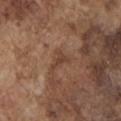<tbp_lesion>
  <biopsy_status>not biopsied; imaged during a skin examination</biopsy_status>
  <lesion_size>
    <long_diameter_mm_approx>3.0</long_diameter_mm_approx>
  </lesion_size>
  <lighting>white-light</lighting>
  <image>
    <source>total-body photography crop</source>
    <field_of_view_mm>15</field_of_view_mm>
  </image>
  <site>left upper arm</site>
  <patient>
    <sex>male</sex>
    <age_approx>75</age_approx>
  </patient>
</tbp_lesion>